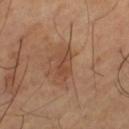{
  "biopsy_status": "not biopsied; imaged during a skin examination",
  "image": {
    "source": "total-body photography crop",
    "field_of_view_mm": 15
  },
  "lighting": "cross-polarized",
  "site": "left thigh",
  "automated_metrics": {
    "shape_asymmetry": 0.3,
    "cielab_L": 45,
    "cielab_a": 21,
    "cielab_b": 30,
    "vs_skin_contrast_norm": 6.0
  },
  "patient": {
    "sex": "male",
    "age_approx": 65
  }
}